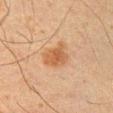The lesion was tiled from a total-body skin photograph and was not biopsied. This is a cross-polarized tile. A male patient, aged around 35. The lesion is on the arm. About 4 mm across. A 15 mm close-up extracted from a 3D total-body photography capture. An algorithmic analysis of the crop reported a lesion area of about 8 mm² and a shape eccentricity near 0.65. And it measured an average lesion color of about L≈45 a*≈19 b*≈31 (CIELAB), about 8 CIELAB-L* units darker than the surrounding skin, and a normalized lesion–skin contrast near 7. It also reported a border-irregularity index near 3.5/10, a color-variation rating of about 2.5/10, and peripheral color asymmetry of about 1. The analysis additionally found an automated nevus-likeness rating near 90 out of 100 and lesion-presence confidence of about 100/100.